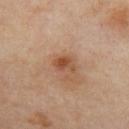| feature | finding |
|---|---|
| biopsy status | catalogued during a skin exam; not biopsied |
| subject | female, aged approximately 50 |
| imaging modality | total-body-photography crop, ~15 mm field of view |
| site | the front of the torso |